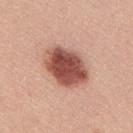Part of a total-body skin-imaging series; this lesion was reviewed on a skin check and was not flagged for biopsy.
A male subject aged around 25.
The lesion is located on the mid back.
Approximately 6 mm at its widest.
This image is a 15 mm lesion crop taken from a total-body photograph.